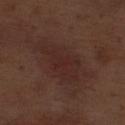| feature | finding |
|---|---|
| follow-up | no biopsy performed (imaged during a skin exam) |